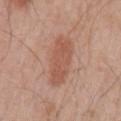- biopsy status: catalogued during a skin exam; not biopsied
- patient: male, aged 68–72
- imaging modality: total-body-photography crop, ~15 mm field of view
- site: the left upper arm